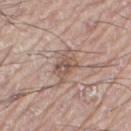workup = catalogued during a skin exam; not biopsied
image = total-body-photography crop, ~15 mm field of view
location = the left leg
tile lighting = white-light illumination
patient = male, roughly 70 years of age